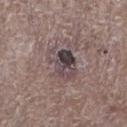follow-up = total-body-photography surveillance lesion; no biopsy
automated lesion analysis = a footprint of about 12 mm², a shape eccentricity near 0.65, and two-axis asymmetry of about 0.3
subject = male, aged 68 to 72
lesion size = ≈4.5 mm
illumination = white-light
image source = 15 mm crop, total-body photography
location = the right lower leg